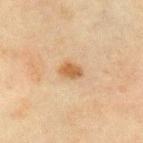Impression:
This lesion was catalogued during total-body skin photography and was not selected for biopsy.
Context:
A male patient about 70 years old. An algorithmic analysis of the crop reported an area of roughly 4 mm², an eccentricity of roughly 0.7, and a symmetry-axis asymmetry near 0.25. The software also gave about 10 CIELAB-L* units darker than the surrounding skin and a lesion-to-skin contrast of about 8 (normalized; higher = more distinct). It also reported a within-lesion color-variation index near 1.5/10 and peripheral color asymmetry of about 0.5. The lesion is located on the front of the torso. A lesion tile, about 15 mm wide, cut from a 3D total-body photograph. Measured at roughly 2.5 mm in maximum diameter. Imaged with cross-polarized lighting.